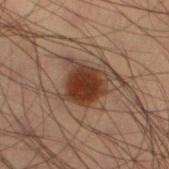{"site": "left lower leg", "lighting": "cross-polarized", "patient": {"sex": "male", "age_approx": 55}, "image": {"source": "total-body photography crop", "field_of_view_mm": 15}, "lesion_size": {"long_diameter_mm_approx": 4.5}, "automated_metrics": {"area_mm2_approx": 13.0, "eccentricity": 0.3, "shape_asymmetry": 0.35, "nevus_likeness_0_100": 100}}